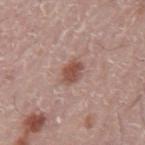This lesion was catalogued during total-body skin photography and was not selected for biopsy. The lesion-visualizer software estimated an area of roughly 5 mm², an outline eccentricity of about 0.75 (0 = round, 1 = elongated), and a symmetry-axis asymmetry near 0.15. The software also gave an average lesion color of about L≈50 a*≈22 b*≈25 (CIELAB), about 12 CIELAB-L* units darker than the surrounding skin, and a normalized border contrast of about 8.5. And it measured border irregularity of about 1.5 on a 0–10 scale, a within-lesion color-variation index near 3/10, and peripheral color asymmetry of about 1. The recorded lesion diameter is about 3 mm. A region of skin cropped from a whole-body photographic capture, roughly 15 mm wide. A male patient, roughly 75 years of age. Located on the mid back. The tile uses white-light illumination.